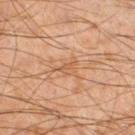Clinical impression:
Part of a total-body skin-imaging series; this lesion was reviewed on a skin check and was not flagged for biopsy.
Clinical summary:
The lesion is on the left lower leg. This image is a 15 mm lesion crop taken from a total-body photograph. A male patient approximately 45 years of age. About 3 mm across.